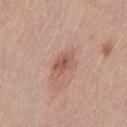| field | value |
|---|---|
| biopsy status | catalogued during a skin exam; not biopsied |
| size | ≈2.5 mm |
| automated metrics | roughly 10 lightness units darker than nearby skin and a lesion-to-skin contrast of about 7 (normalized; higher = more distinct); peripheral color asymmetry of about 1 |
| subject | female, approximately 40 years of age |
| image | 15 mm crop, total-body photography |
| site | the left thigh |